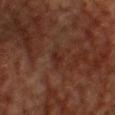Assessment:
This lesion was catalogued during total-body skin photography and was not selected for biopsy.
Clinical summary:
A male subject, aged around 60. On the chest. The tile uses cross-polarized illumination. A region of skin cropped from a whole-body photographic capture, roughly 15 mm wide.